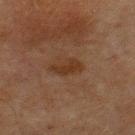Case summary:
– notes — no biopsy performed (imaged during a skin exam)
– tile lighting — cross-polarized
– patient — male, aged 63–67
– site — the chest
– acquisition — ~15 mm crop, total-body skin-cancer survey
– image-analysis metrics — a border-irregularity index near 3/10, internal color variation of about 1.5 on a 0–10 scale, and peripheral color asymmetry of about 0.5; a nevus-likeness score of about 45/100 and a detector confidence of about 100 out of 100 that the crop contains a lesion
– lesion diameter — ~3.5 mm (longest diameter)